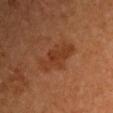| field | value |
|---|---|
| workup | total-body-photography surveillance lesion; no biopsy |
| patient | male, in their mid- to late 50s |
| image | ~15 mm tile from a whole-body skin photo |
| illumination | cross-polarized illumination |
| automated metrics | a footprint of about 10 mm², an outline eccentricity of about 0.85 (0 = round, 1 = elongated), and a shape-asymmetry score of about 0.25 (0 = symmetric); a mean CIELAB color near L≈34 a*≈23 b*≈31, about 7 CIELAB-L* units darker than the surrounding skin, and a normalized lesion–skin contrast near 6.5; a border-irregularity index near 4/10 and a color-variation rating of about 2/10; an automated nevus-likeness rating near 25 out of 100 and a lesion-detection confidence of about 100/100 |
| size | ≈4.5 mm |
| anatomic site | the left upper arm |